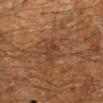Captured during whole-body skin photography for melanoma surveillance; the lesion was not biopsied. From the leg. The lesion's longest dimension is about 2.5 mm. A close-up tile cropped from a whole-body skin photograph, about 15 mm across. This is a cross-polarized tile. A male subject, aged around 60.